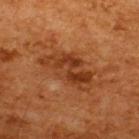{"biopsy_status": "not biopsied; imaged during a skin examination", "lighting": "cross-polarized", "site": "upper back", "image": {"source": "total-body photography crop", "field_of_view_mm": 15}, "patient": {"sex": "male", "age_approx": 65}}